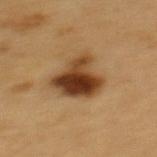The lesion was photographed on a routine skin check and not biopsied; there is no pathology result. A region of skin cropped from a whole-body photographic capture, roughly 15 mm wide. The tile uses cross-polarized illumination. The lesion is located on the mid back. Longest diameter approximately 5 mm. The patient is a male approximately 60 years of age.